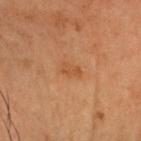<tbp_lesion>
<biopsy_status>not biopsied; imaged during a skin examination</biopsy_status>
<image>
  <source>total-body photography crop</source>
  <field_of_view_mm>15</field_of_view_mm>
</image>
<lighting>cross-polarized</lighting>
<lesion_size>
  <long_diameter_mm_approx>2.5</long_diameter_mm_approx>
</lesion_size>
<patient>
  <sex>male</sex>
  <age_approx>55</age_approx>
</patient>
<site>head or neck</site>
</tbp_lesion>